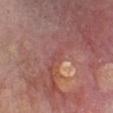Captured during whole-body skin photography for melanoma surveillance; the lesion was not biopsied.
On the chest.
This is a white-light tile.
About 2 mm across.
A 15 mm close-up tile from a total-body photography series done for melanoma screening.
A male subject about 65 years old.